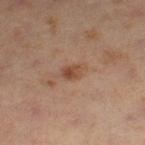The lesion is located on the leg. This is a cross-polarized tile. Longest diameter approximately 3 mm. A male subject, roughly 60 years of age. A 15 mm close-up tile from a total-body photography series done for melanoma screening. Automated image analysis of the tile measured a peripheral color-asymmetry measure near 1.5. The analysis additionally found a lesion-detection confidence of about 100/100.